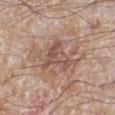Assessment:
The lesion was tiled from a total-body skin photograph and was not biopsied.
Background:
The lesion is on the left lower leg. A region of skin cropped from a whole-body photographic capture, roughly 15 mm wide. The subject is a male roughly 60 years of age.